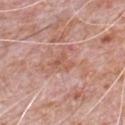notes = catalogued during a skin exam; not biopsied | image = 15 mm crop, total-body photography | anatomic site = the chest | subject = male, in their 80s | lesion diameter = ≈2.5 mm | illumination = white-light | image-analysis metrics = an area of roughly 4 mm², a shape eccentricity near 0.6, and a symmetry-axis asymmetry near 0.5; a border-irregularity rating of about 5/10 and radial color variation of about 1; a nevus-likeness score of about 0/100 and lesion-presence confidence of about 90/100.